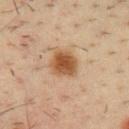This lesion was catalogued during total-body skin photography and was not selected for biopsy. The lesion's longest dimension is about 3 mm. An algorithmic analysis of the crop reported a color-variation rating of about 3.5/10 and a peripheral color-asymmetry measure near 1. It also reported a nevus-likeness score of about 100/100 and lesion-presence confidence of about 100/100. The subject is a male aged approximately 35. The lesion is located on the chest. This is a cross-polarized tile. Cropped from a whole-body photographic skin survey; the tile spans about 15 mm.